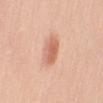notes: no biopsy performed (imaged during a skin exam)
illumination: white-light illumination
image-analysis metrics: an automated nevus-likeness rating near 95 out of 100 and lesion-presence confidence of about 100/100
acquisition: ~15 mm crop, total-body skin-cancer survey
patient: female, about 50 years old
anatomic site: the right upper arm
diameter: ≈3 mm